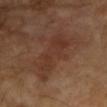The lesion was tiled from a total-body skin photograph and was not biopsied.
A lesion tile, about 15 mm wide, cut from a 3D total-body photograph.
A female patient, roughly 70 years of age.
The lesion is located on the left forearm.